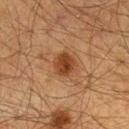Part of a total-body skin-imaging series; this lesion was reviewed on a skin check and was not flagged for biopsy. A male subject, roughly 60 years of age. The lesion is located on the chest. Cropped from a whole-body photographic skin survey; the tile spans about 15 mm.